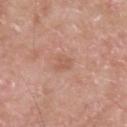| key | value |
|---|---|
| notes | total-body-photography surveillance lesion; no biopsy |
| lighting | white-light illumination |
| diameter | ≈2.5 mm |
| site | the back |
| patient | male, in their mid- to late 60s |
| image source | ~15 mm crop, total-body skin-cancer survey |
| automated lesion analysis | a mean CIELAB color near L≈58 a*≈22 b*≈29 and a normalized border contrast of about 4.5; a nevus-likeness score of about 0/100 and lesion-presence confidence of about 100/100 |